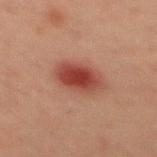biopsy status: no biopsy performed (imaged during a skin exam) | body site: the mid back | automated metrics: a mean CIELAB color near L≈36 a*≈25 b*≈24, about 11 CIELAB-L* units darker than the surrounding skin, and a lesion-to-skin contrast of about 10 (normalized; higher = more distinct); a nevus-likeness score of about 100/100 | lesion diameter: ~4.5 mm (longest diameter) | image source: ~15 mm crop, total-body skin-cancer survey | subject: male, in their mid- to late 40s.